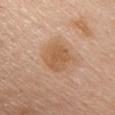Q: What is the imaging modality?
A: total-body-photography crop, ~15 mm field of view
Q: Who is the patient?
A: male, aged 58–62
Q: Where on the body is the lesion?
A: the front of the torso